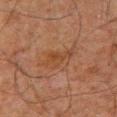* follow-up — catalogued during a skin exam; not biopsied
* imaging modality — ~15 mm tile from a whole-body skin photo
* lesion size — ≈4 mm
* subject — male, approximately 60 years of age
* location — the chest
* illumination — cross-polarized illumination